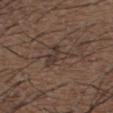Part of a total-body skin-imaging series; this lesion was reviewed on a skin check and was not flagged for biopsy. The lesion is located on the upper back. Measured at roughly 4.5 mm in maximum diameter. A lesion tile, about 15 mm wide, cut from a 3D total-body photograph. A male patient aged approximately 50.